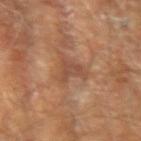The lesion was photographed on a routine skin check and not biopsied; there is no pathology result. A 15 mm crop from a total-body photograph taken for skin-cancer surveillance. From the left upper arm. A male subject roughly 65 years of age. This is a cross-polarized tile. Measured at roughly 4 mm in maximum diameter. Automated image analysis of the tile measured a lesion area of about 7.5 mm², an outline eccentricity of about 0.4 (0 = round, 1 = elongated), and a shape-asymmetry score of about 0.6 (0 = symmetric). It also reported lesion-presence confidence of about 90/100.